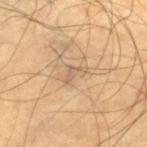| field | value |
|---|---|
| acquisition | total-body-photography crop, ~15 mm field of view |
| patient | male, about 65 years old |
| location | the leg |